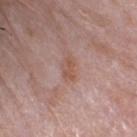| field | value |
|---|---|
| follow-up | total-body-photography surveillance lesion; no biopsy |
| lesion size | about 3 mm |
| subject | female, aged approximately 85 or older |
| tile lighting | white-light illumination |
| image source | total-body-photography crop, ~15 mm field of view |
| location | the head or neck |